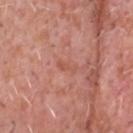{"biopsy_status": "not biopsied; imaged during a skin examination"}